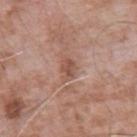Clinical impression:
Part of a total-body skin-imaging series; this lesion was reviewed on a skin check and was not flagged for biopsy.
Image and clinical context:
This is a white-light tile. A region of skin cropped from a whole-body photographic capture, roughly 15 mm wide. Longest diameter approximately 2.5 mm. The lesion is on the left upper arm. The subject is a male aged around 75.